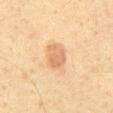Recorded during total-body skin imaging; not selected for excision or biopsy. On the abdomen. About 3 mm across. A male subject in their 60s. The lesion-visualizer software estimated an area of roughly 7.5 mm² and an eccentricity of roughly 0.55. The analysis additionally found a mean CIELAB color near L≈57 a*≈18 b*≈33, a lesion–skin lightness drop of about 8, and a normalized lesion–skin contrast near 6. It also reported border irregularity of about 1.5 on a 0–10 scale and a within-lesion color-variation index near 2.5/10. A lesion tile, about 15 mm wide, cut from a 3D total-body photograph.